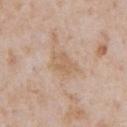follow-up: total-body-photography surveillance lesion; no biopsy | image source: total-body-photography crop, ~15 mm field of view | tile lighting: white-light illumination | diameter: ~3.5 mm (longest diameter) | location: the chest | automated lesion analysis: a nevus-likeness score of about 0/100 and a detector confidence of about 100 out of 100 that the crop contains a lesion | subject: male, about 65 years old.